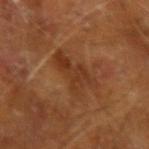Clinical impression: Imaged during a routine full-body skin examination; the lesion was not biopsied and no histopathology is available. Acquisition and patient details: The subject is a male aged 63 to 67. A lesion tile, about 15 mm wide, cut from a 3D total-body photograph. Measured at roughly 6 mm in maximum diameter. Located on the arm. Imaged with cross-polarized lighting.